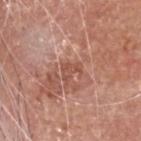biopsy status = no biopsy performed (imaged during a skin exam) | lesion diameter = ≈2.5 mm | anatomic site = the head or neck | automated metrics = border irregularity of about 5.5 on a 0–10 scale and a within-lesion color-variation index near 1/10 | image = total-body-photography crop, ~15 mm field of view | patient = male, approximately 80 years of age | illumination = white-light.